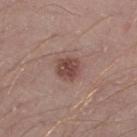Q: Was a biopsy performed?
A: imaged on a skin check; not biopsied
Q: Who is the patient?
A: male, aged 38–42
Q: How large is the lesion?
A: about 2.5 mm
Q: Automated lesion metrics?
A: an area of roughly 6 mm² and a shape-asymmetry score of about 0.2 (0 = symmetric); an average lesion color of about L≈45 a*≈21 b*≈23 (CIELAB) and about 11 CIELAB-L* units darker than the surrounding skin; border irregularity of about 2 on a 0–10 scale, internal color variation of about 2.5 on a 0–10 scale, and radial color variation of about 1; a classifier nevus-likeness of about 80/100 and lesion-presence confidence of about 100/100
Q: How was this image acquired?
A: total-body-photography crop, ~15 mm field of view
Q: Where on the body is the lesion?
A: the right thigh